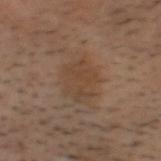<tbp_lesion>
<biopsy_status>not biopsied; imaged during a skin examination</biopsy_status>
</tbp_lesion>